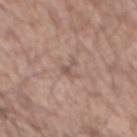| field | value |
|---|---|
| follow-up | no biopsy performed (imaged during a skin exam) |
| imaging modality | total-body-photography crop, ~15 mm field of view |
| subject | male, roughly 55 years of age |
| lesion diameter | ~2.5 mm (longest diameter) |
| anatomic site | the mid back |